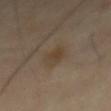The recorded lesion diameter is about 3.5 mm. The lesion is on the mid back. The subject is a male roughly 65 years of age. A lesion tile, about 15 mm wide, cut from a 3D total-body photograph. Automated image analysis of the tile measured a lesion area of about 5 mm² and an eccentricity of roughly 0.8. It also reported a classifier nevus-likeness of about 25/100 and a lesion-detection confidence of about 100/100.